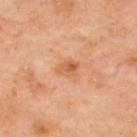No biopsy was performed on this lesion — it was imaged during a full skin examination and was not determined to be concerning.
Approximately 2.5 mm at its widest.
A female subject about 60 years old.
The lesion-visualizer software estimated a lesion color around L≈60 a*≈26 b*≈40 in CIELAB, roughly 9 lightness units darker than nearby skin, and a normalized border contrast of about 6.5. The software also gave border irregularity of about 2 on a 0–10 scale, a within-lesion color-variation index near 5/10, and a peripheral color-asymmetry measure near 2. The software also gave a nevus-likeness score of about 0/100 and lesion-presence confidence of about 100/100.
A close-up tile cropped from a whole-body skin photograph, about 15 mm across.
The lesion is on the back.
Imaged with cross-polarized lighting.